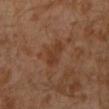Located on the left lower leg. An algorithmic analysis of the crop reported an outline eccentricity of about 0.9 (0 = round, 1 = elongated). And it measured a within-lesion color-variation index near 2/10 and peripheral color asymmetry of about 0.5. A male subject, aged approximately 30. Captured under cross-polarized illumination. Cropped from a total-body skin-imaging series; the visible field is about 15 mm. Longest diameter approximately 4.5 mm.